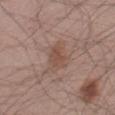biopsy status: catalogued during a skin exam; not biopsied | automated metrics: a classifier nevus-likeness of about 10/100 and a detector confidence of about 100 out of 100 that the crop contains a lesion | patient: male, aged 43 to 47 | lesion size: ~3 mm (longest diameter) | site: the right thigh | image: total-body-photography crop, ~15 mm field of view.